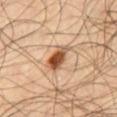Notes:
* workup: no biopsy performed (imaged during a skin exam)